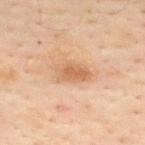Q: Is there a histopathology result?
A: catalogued during a skin exam; not biopsied
Q: Illumination type?
A: cross-polarized illumination
Q: Patient demographics?
A: male, approximately 50 years of age
Q: Automated lesion metrics?
A: an outline eccentricity of about 0.85 (0 = round, 1 = elongated) and two-axis asymmetry of about 0.25; a nevus-likeness score of about 25/100 and lesion-presence confidence of about 100/100
Q: What is the imaging modality?
A: ~15 mm crop, total-body skin-cancer survey
Q: How large is the lesion?
A: ~4 mm (longest diameter)
Q: Where on the body is the lesion?
A: the back